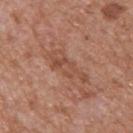{"biopsy_status": "not biopsied; imaged during a skin examination", "patient": {"sex": "male", "age_approx": 65}, "site": "upper back", "automated_metrics": {"area_mm2_approx": 8.0, "eccentricity": 0.95, "nevus_likeness_0_100": 0, "lesion_detection_confidence_0_100": 100}, "image": {"source": "total-body photography crop", "field_of_view_mm": 15}}